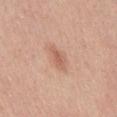Impression:
No biopsy was performed on this lesion — it was imaged during a full skin examination and was not determined to be concerning.
Background:
The patient is a female aged 38–42. The lesion is located on the abdomen. Cropped from a total-body skin-imaging series; the visible field is about 15 mm. The lesion-visualizer software estimated a normalized border contrast of about 6. And it measured a classifier nevus-likeness of about 50/100 and lesion-presence confidence of about 100/100. Captured under white-light illumination. The recorded lesion diameter is about 3 mm.